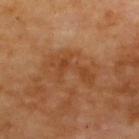notes = imaged on a skin check; not biopsied | body site = the upper back | diameter = ~5.5 mm (longest diameter) | TBP lesion metrics = an area of roughly 12 mm², a shape eccentricity near 0.85, and two-axis asymmetry of about 0.55; an automated nevus-likeness rating near 0 out of 100 and lesion-presence confidence of about 100/100 | image = total-body-photography crop, ~15 mm field of view | subject = in their mid- to late 60s.